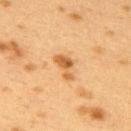Clinical impression: Part of a total-body skin-imaging series; this lesion was reviewed on a skin check and was not flagged for biopsy. Image and clinical context: The subject is a female aged around 40. This is a cross-polarized tile. A lesion tile, about 15 mm wide, cut from a 3D total-body photograph. From the back.